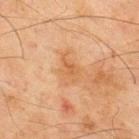biopsy status: imaged on a skin check; not biopsied
diameter: ~3 mm (longest diameter)
illumination: cross-polarized illumination
location: the upper back
imaging modality: ~15 mm tile from a whole-body skin photo
patient: male, roughly 45 years of age